Located on the leg. This image is a 15 mm lesion crop taken from a total-body photograph. A male subject, about 80 years old:
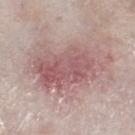On biopsy, histopathology showed a superficial basal cell carcinoma.